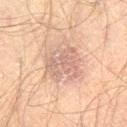The lesion was photographed on a routine skin check and not biopsied; there is no pathology result. A male patient, aged approximately 60. Imaged with cross-polarized lighting. A 15 mm close-up extracted from a 3D total-body photography capture. The lesion is located on the right thigh. Longest diameter approximately 4.5 mm.